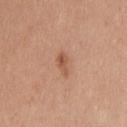{"site": "upper back", "automated_metrics": {"vs_skin_darker_L": 10.0, "vs_skin_contrast_norm": 7.0, "border_irregularity_0_10": 3.5, "peripheral_color_asymmetry": 1.0, "nevus_likeness_0_100": 70, "lesion_detection_confidence_0_100": 100}, "patient": {"sex": "male", "age_approx": 35}, "image": {"source": "total-body photography crop", "field_of_view_mm": 15}, "lesion_size": {"long_diameter_mm_approx": 2.5}, "lighting": "white-light"}